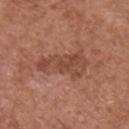Located on the chest. This image is a 15 mm lesion crop taken from a total-body photograph. The lesion-visualizer software estimated a lesion area of about 14 mm² and a shape eccentricity near 0.9. The software also gave a classifier nevus-likeness of about 0/100 and lesion-presence confidence of about 100/100. The tile uses white-light illumination. A male subject, aged 73 to 77. Measured at roughly 6.5 mm in maximum diameter.